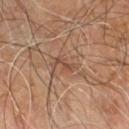Imaged during a routine full-body skin examination; the lesion was not biopsied and no histopathology is available.
Cropped from a total-body skin-imaging series; the visible field is about 15 mm.
Measured at roughly 3 mm in maximum diameter.
The tile uses cross-polarized illumination.
The lesion is located on the right leg.
A male subject aged 58–62.